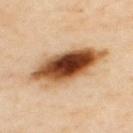Q: Was a biopsy performed?
A: imaged on a skin check; not biopsied